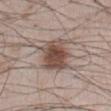workup = total-body-photography surveillance lesion; no biopsy | anatomic site = the front of the torso | tile lighting = white-light illumination | patient = male, roughly 50 years of age | acquisition = ~15 mm tile from a whole-body skin photo.